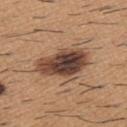The lesion was tiled from a total-body skin photograph and was not biopsied.
Automated image analysis of the tile measured an area of roughly 19 mm² and a shape-asymmetry score of about 0.2 (0 = symmetric). And it measured an average lesion color of about L≈44 a*≈19 b*≈27 (CIELAB) and a normalized border contrast of about 12.5. And it measured an automated nevus-likeness rating near 75 out of 100 and a detector confidence of about 100 out of 100 that the crop contains a lesion.
Imaged with white-light lighting.
Longest diameter approximately 6.5 mm.
A 15 mm close-up extracted from a 3D total-body photography capture.
From the upper back.
A male patient approximately 60 years of age.